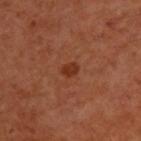Assessment:
Part of a total-body skin-imaging series; this lesion was reviewed on a skin check and was not flagged for biopsy.
Acquisition and patient details:
The lesion-visualizer software estimated a mean CIELAB color near L≈32 a*≈26 b*≈32, a lesion–skin lightness drop of about 8, and a lesion-to-skin contrast of about 8 (normalized; higher = more distinct). The analysis additionally found a border-irregularity rating of about 2/10 and a within-lesion color-variation index near 1.5/10. The software also gave a classifier nevus-likeness of about 90/100 and a lesion-detection confidence of about 100/100. A lesion tile, about 15 mm wide, cut from a 3D total-body photograph. The tile uses cross-polarized illumination. Approximately 2 mm at its widest. A male patient, about 55 years old. The lesion is on the upper back.